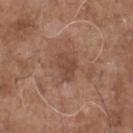Impression: The lesion was tiled from a total-body skin photograph and was not biopsied. Image and clinical context: Located on the chest. A close-up tile cropped from a whole-body skin photograph, about 15 mm across. A male patient about 70 years old. Automated tile analysis of the lesion measured a footprint of about 5 mm², a shape eccentricity near 0.8, and two-axis asymmetry of about 0.4. The analysis additionally found a border-irregularity rating of about 4/10, a within-lesion color-variation index near 2/10, and peripheral color asymmetry of about 0.5. It also reported an automated nevus-likeness rating near 0 out of 100. Measured at roughly 3 mm in maximum diameter.